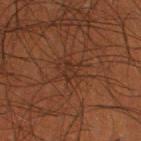Assessment: Recorded during total-body skin imaging; not selected for excision or biopsy. Acquisition and patient details: Measured at roughly 2.5 mm in maximum diameter. The lesion is on the right lower leg. A region of skin cropped from a whole-body photographic capture, roughly 15 mm wide. A male patient, about 50 years old.